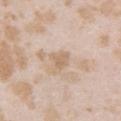{"biopsy_status": "not biopsied; imaged during a skin examination", "automated_metrics": {"border_irregularity_0_10": 3.0, "color_variation_0_10": 1.5, "peripheral_color_asymmetry": 0.5, "nevus_likeness_0_100": 0}, "lighting": "white-light", "image": {"source": "total-body photography crop", "field_of_view_mm": 15}, "site": "arm", "patient": {"sex": "female", "age_approx": 25}}